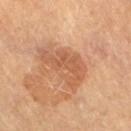Acquisition and patient details: Captured under cross-polarized illumination. An algorithmic analysis of the crop reported a lesion area of about 14 mm² and a shape eccentricity near 0.85. And it measured a lesion color around L≈55 a*≈23 b*≈34 in CIELAB, about 8 CIELAB-L* units darker than the surrounding skin, and a lesion-to-skin contrast of about 5.5 (normalized; higher = more distinct). The software also gave an automated nevus-likeness rating near 0 out of 100 and lesion-presence confidence of about 100/100. The subject is a female roughly 70 years of age. The lesion is on the right thigh. A lesion tile, about 15 mm wide, cut from a 3D total-body photograph.